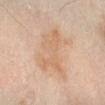follow-up = no biopsy performed (imaged during a skin exam); image = 15 mm crop, total-body photography; tile lighting = cross-polarized; site = the right forearm; subject = male, roughly 55 years of age.